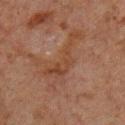Impression:
Recorded during total-body skin imaging; not selected for excision or biopsy.